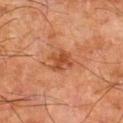Impression: The lesion was tiled from a total-body skin photograph and was not biopsied. Clinical summary: From the left thigh. Captured under cross-polarized illumination. The lesion's longest dimension is about 3 mm. Cropped from a total-body skin-imaging series; the visible field is about 15 mm. A male patient, aged 78 to 82. An algorithmic analysis of the crop reported a border-irregularity rating of about 2.5/10 and a within-lesion color-variation index near 3.5/10.